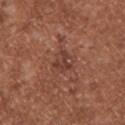follow-up = no biopsy performed (imaged during a skin exam) | imaging modality = ~15 mm crop, total-body skin-cancer survey | lighting = white-light illumination | anatomic site = the upper back | TBP lesion metrics = an average lesion color of about L≈39 a*≈24 b*≈26 (CIELAB) and a lesion–skin lightness drop of about 7; a border-irregularity rating of about 4/10, internal color variation of about 2.5 on a 0–10 scale, and a peripheral color-asymmetry measure near 1; a classifier nevus-likeness of about 0/100 | lesion diameter = ~2.5 mm (longest diameter) | patient = male, in their mid-40s.